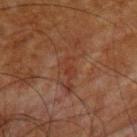A roughly 15 mm field-of-view crop from a total-body skin photograph. The lesion's longest dimension is about 2.5 mm. The lesion is on the upper back. The tile uses cross-polarized illumination. A male subject aged approximately 65.